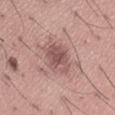Clinical impression: Imaged during a routine full-body skin examination; the lesion was not biopsied and no histopathology is available. Context: Automated tile analysis of the lesion measured an area of roughly 8.5 mm², a shape eccentricity near 0.45, and a symmetry-axis asymmetry near 0.25. And it measured roughly 10 lightness units darker than nearby skin and a normalized border contrast of about 7. And it measured a border-irregularity index near 3.5/10, internal color variation of about 3 on a 0–10 scale, and peripheral color asymmetry of about 1. It also reported a nevus-likeness score of about 25/100. The lesion is on the lower back. A male subject, in their mid-40s. The lesion's longest dimension is about 3.5 mm. A roughly 15 mm field-of-view crop from a total-body skin photograph.